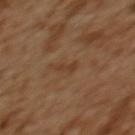Clinical impression:
Imaged during a routine full-body skin examination; the lesion was not biopsied and no histopathology is available.
Background:
A female subject, approximately 55 years of age. A roughly 15 mm field-of-view crop from a total-body skin photograph. Located on the upper back.